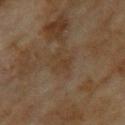Assessment: This lesion was catalogued during total-body skin photography and was not selected for biopsy.